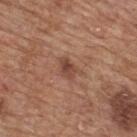The lesion was tiled from a total-body skin photograph and was not biopsied.
The lesion is located on the upper back.
A male patient, aged 63 to 67.
Cropped from a whole-body photographic skin survey; the tile spans about 15 mm.
This is a white-light tile.
About 3 mm across.